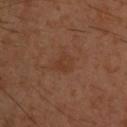biopsy status: imaged on a skin check; not biopsied | acquisition: 15 mm crop, total-body photography | anatomic site: the upper back | patient: male, aged approximately 30 | lighting: cross-polarized | size: ≈2.5 mm.